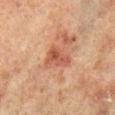Impression:
This lesion was catalogued during total-body skin photography and was not selected for biopsy.
Image and clinical context:
About 4 mm across. On the right lower leg. A male patient, aged around 70. This is a cross-polarized tile. A region of skin cropped from a whole-body photographic capture, roughly 15 mm wide.